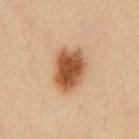workup = imaged on a skin check; not biopsied
patient = male, in their mid-30s
image = 15 mm crop, total-body photography
TBP lesion metrics = a lesion area of about 14 mm² and a symmetry-axis asymmetry near 0.2; an automated nevus-likeness rating near 100 out of 100 and lesion-presence confidence of about 100/100
anatomic site = the chest